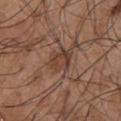This lesion was catalogued during total-body skin photography and was not selected for biopsy. A male patient aged approximately 55. Automated tile analysis of the lesion measured a lesion area of about 6.5 mm² and a symmetry-axis asymmetry near 0.25. The software also gave about 8 CIELAB-L* units darker than the surrounding skin and a normalized lesion–skin contrast near 6.5. The tile uses white-light illumination. The lesion's longest dimension is about 3 mm. A lesion tile, about 15 mm wide, cut from a 3D total-body photograph. The lesion is located on the front of the torso.